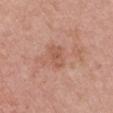Findings:
* follow-up — no biopsy performed (imaged during a skin exam)
* acquisition — ~15 mm tile from a whole-body skin photo
* size — ≈3 mm
* tile lighting — white-light
* body site — the left upper arm
* patient — female, approximately 45 years of age